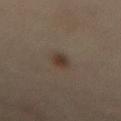  biopsy_status: not biopsied; imaged during a skin examination
  patient:
    sex: female
    age_approx: 60
  image:
    source: total-body photography crop
    field_of_view_mm: 15
  lesion_size:
    long_diameter_mm_approx: 3.0
  site: lower back
  automated_metrics:
    eccentricity: 0.8
    shape_asymmetry: 0.2
    cielab_L: 30
    cielab_a: 11
    cielab_b: 20
    vs_skin_darker_L: 7.0
    vs_skin_contrast_norm: 8.0
    nevus_likeness_0_100: 95
    lesion_detection_confidence_0_100: 100